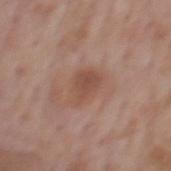Clinical impression:
Imaged during a routine full-body skin examination; the lesion was not biopsied and no histopathology is available.
Image and clinical context:
The tile uses white-light illumination. A 15 mm close-up tile from a total-body photography series done for melanoma screening. About 3.5 mm across. The lesion is located on the back. The total-body-photography lesion software estimated a mean CIELAB color near L≈49 a*≈21 b*≈27 and a lesion–skin lightness drop of about 8. A male patient, aged approximately 75.